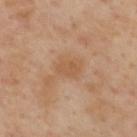{"biopsy_status": "not biopsied; imaged during a skin examination", "site": "back", "lighting": "cross-polarized", "automated_metrics": {"area_mm2_approx": 4.0, "eccentricity": 0.7, "shape_asymmetry": 0.25, "cielab_L": 54, "cielab_a": 20, "cielab_b": 35, "vs_skin_contrast_norm": 5.5, "border_irregularity_0_10": 2.5, "color_variation_0_10": 1.5, "peripheral_color_asymmetry": 0.5, "nevus_likeness_0_100": 0, "lesion_detection_confidence_0_100": 100}, "lesion_size": {"long_diameter_mm_approx": 2.5}, "patient": {"sex": "male", "age_approx": 55}, "image": {"source": "total-body photography crop", "field_of_view_mm": 15}}